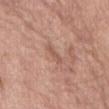The lesion was tiled from a total-body skin photograph and was not biopsied.
From the front of the torso.
Automated tile analysis of the lesion measured a footprint of about 3 mm², an outline eccentricity of about 0.95 (0 = round, 1 = elongated), and a shape-asymmetry score of about 0.4 (0 = symmetric). The software also gave an average lesion color of about L≈56 a*≈21 b*≈28 (CIELAB) and a normalized border contrast of about 5. The analysis additionally found a border-irregularity rating of about 4.5/10, internal color variation of about 0 on a 0–10 scale, and radial color variation of about 0.
Approximately 3 mm at its widest.
Imaged with white-light lighting.
A male patient, about 70 years old.
A region of skin cropped from a whole-body photographic capture, roughly 15 mm wide.